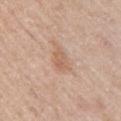Captured during whole-body skin photography for melanoma surveillance; the lesion was not biopsied.
The lesion is located on the right upper arm.
Measured at roughly 2.5 mm in maximum diameter.
A 15 mm close-up tile from a total-body photography series done for melanoma screening.
Automated tile analysis of the lesion measured a lesion area of about 4 mm², an eccentricity of roughly 0.6, and two-axis asymmetry of about 0.25. The analysis additionally found an average lesion color of about L≈61 a*≈19 b*≈31 (CIELAB), a lesion–skin lightness drop of about 8, and a lesion-to-skin contrast of about 6 (normalized; higher = more distinct).
A female patient, roughly 50 years of age.
The tile uses white-light illumination.